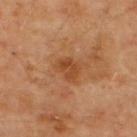{
  "biopsy_status": "not biopsied; imaged during a skin examination",
  "lesion_size": {
    "long_diameter_mm_approx": 3.0
  },
  "automated_metrics": {
    "area_mm2_approx": 6.5,
    "eccentricity": 0.6,
    "shape_asymmetry": 0.3,
    "border_irregularity_0_10": 3.0,
    "color_variation_0_10": 3.0,
    "lesion_detection_confidence_0_100": 100
  },
  "site": "upper back",
  "patient": {
    "sex": "male",
    "age_approx": 65
  },
  "image": {
    "source": "total-body photography crop",
    "field_of_view_mm": 15
  }
}